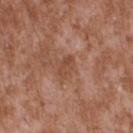Clinical impression: Captured during whole-body skin photography for melanoma surveillance; the lesion was not biopsied. Image and clinical context: Cropped from a total-body skin-imaging series; the visible field is about 15 mm. A male patient in their mid-40s. Located on the back. Imaged with white-light lighting.